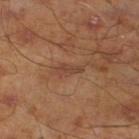This lesion was catalogued during total-body skin photography and was not selected for biopsy.
A 15 mm close-up extracted from a 3D total-body photography capture.
Located on the left lower leg.
A male subject, roughly 45 years of age.
Imaged with cross-polarized lighting.